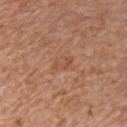Located on the chest. The patient is a male about 65 years old. A lesion tile, about 15 mm wide, cut from a 3D total-body photograph.